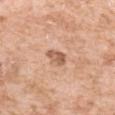{
  "patient": {
    "sex": "female",
    "age_approx": 40
  },
  "site": "left upper arm",
  "automated_metrics": {
    "area_mm2_approx": 3.5,
    "eccentricity": 0.85,
    "shape_asymmetry": 0.35,
    "vs_skin_darker_L": 12.0,
    "nevus_likeness_0_100": 20,
    "lesion_detection_confidence_0_100": 100
  },
  "lesion_size": {
    "long_diameter_mm_approx": 3.0
  },
  "lighting": "white-light",
  "image": {
    "source": "total-body photography crop",
    "field_of_view_mm": 15
  }
}